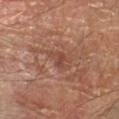  biopsy_status: not biopsied; imaged during a skin examination
  site: left forearm
  lighting: cross-polarized
  automated_metrics:
    cielab_L: 47
    cielab_a: 23
    cielab_b: 28
    vs_skin_darker_L: 7.0
    vs_skin_contrast_norm: 5.5
    nevus_likeness_0_100: 0
    lesion_detection_confidence_0_100: 95
  image:
    source: total-body photography crop
    field_of_view_mm: 15
  lesion_size:
    long_diameter_mm_approx: 3.5